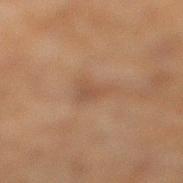Assessment:
Recorded during total-body skin imaging; not selected for excision or biopsy.
Context:
The lesion's longest dimension is about 3 mm. A male patient roughly 70 years of age. Located on the right lower leg. This is a cross-polarized tile. A roughly 15 mm field-of-view crop from a total-body skin photograph.